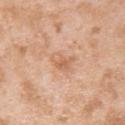• biopsy status · catalogued during a skin exam; not biopsied
• image-analysis metrics · an area of roughly 5 mm², an eccentricity of roughly 0.65, and a shape-asymmetry score of about 0.35 (0 = symmetric); a mean CIELAB color near L≈63 a*≈22 b*≈34, a lesion–skin lightness drop of about 8, and a normalized border contrast of about 5.5; an automated nevus-likeness rating near 0 out of 100
• lesion size · ~3 mm (longest diameter)
• subject · male, aged around 25
• image · ~15 mm crop, total-body skin-cancer survey
• tile lighting · white-light
• site · the upper back